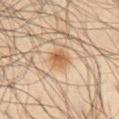Part of a total-body skin-imaging series; this lesion was reviewed on a skin check and was not flagged for biopsy.
A male subject aged around 65.
A close-up tile cropped from a whole-body skin photograph, about 15 mm across.
The lesion-visualizer software estimated roughly 8 lightness units darker than nearby skin and a normalized border contrast of about 7.5. The analysis additionally found a within-lesion color-variation index near 4/10 and a peripheral color-asymmetry measure near 1.5.
Longest diameter approximately 2.5 mm.
On the abdomen.